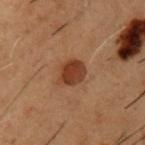{
  "biopsy_status": "not biopsied; imaged during a skin examination",
  "image": {
    "source": "total-body photography crop",
    "field_of_view_mm": 15
  },
  "lesion_size": {
    "long_diameter_mm_approx": 3.0
  },
  "site": "chest",
  "automated_metrics": {
    "cielab_L": 30,
    "cielab_a": 20,
    "cielab_b": 26,
    "peripheral_color_asymmetry": 1.0
  },
  "lighting": "cross-polarized",
  "patient": {
    "sex": "male",
    "age_approx": 50
  }
}